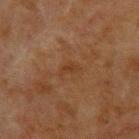Clinical impression:
No biopsy was performed on this lesion — it was imaged during a full skin examination and was not determined to be concerning.
Context:
The lesion is located on the upper back. About 2.5 mm across. The tile uses cross-polarized illumination. A male patient, aged 48–52. This image is a 15 mm lesion crop taken from a total-body photograph. An algorithmic analysis of the crop reported a lesion area of about 2.5 mm², an outline eccentricity of about 0.85 (0 = round, 1 = elongated), and a shape-asymmetry score of about 0.4 (0 = symmetric). It also reported a lesion color around L≈30 a*≈17 b*≈27 in CIELAB, roughly 4 lightness units darker than nearby skin, and a normalized border contrast of about 5. And it measured a detector confidence of about 100 out of 100 that the crop contains a lesion.